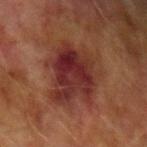Assessment:
Recorded during total-body skin imaging; not selected for excision or biopsy.
Clinical summary:
The recorded lesion diameter is about 7 mm. The tile uses cross-polarized illumination. A 15 mm close-up tile from a total-body photography series done for melanoma screening. From the left upper arm. The subject is a male aged approximately 75.